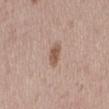Q: Was this lesion biopsied?
A: imaged on a skin check; not biopsied
Q: What is the lesion's diameter?
A: about 2.5 mm
Q: How was this image acquired?
A: ~15 mm tile from a whole-body skin photo
Q: Patient demographics?
A: female, aged 28–32
Q: Lesion location?
A: the right thigh